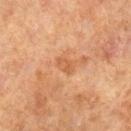workup = total-body-photography surveillance lesion; no biopsy
tile lighting = cross-polarized
imaging modality = ~15 mm tile from a whole-body skin photo
location = the right lower leg
diameter = ≈2.5 mm
patient = male, aged approximately 70